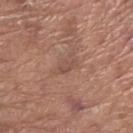  patient:
    sex: male
    age_approx: 80
  image:
    source: total-body photography crop
    field_of_view_mm: 15
  site: arm